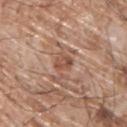image:
  source: total-body photography crop
  field_of_view_mm: 15
patient:
  sex: male
  age_approx: 65
site: back